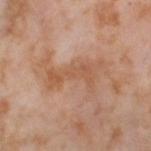| field | value |
|---|---|
| follow-up | no biopsy performed (imaged during a skin exam) |
| image source | total-body-photography crop, ~15 mm field of view |
| subject | female, aged around 55 |
| size | ≈6.5 mm |
| body site | the left thigh |